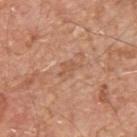Q: Automated lesion metrics?
A: a within-lesion color-variation index near 1/10 and radial color variation of about 0.5
Q: What kind of image is this?
A: ~15 mm crop, total-body skin-cancer survey
Q: Lesion location?
A: the upper back
Q: What are the patient's age and sex?
A: male, in their mid-60s